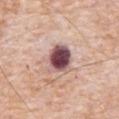Imaged during a routine full-body skin examination; the lesion was not biopsied and no histopathology is available.